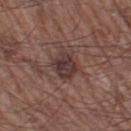Q: Was a biopsy performed?
A: no biopsy performed (imaged during a skin exam)
Q: What is the imaging modality?
A: total-body-photography crop, ~15 mm field of view
Q: What is the anatomic site?
A: the left thigh
Q: What is the lesion's diameter?
A: about 4 mm
Q: What are the patient's age and sex?
A: male, roughly 60 years of age
Q: What lighting was used for the tile?
A: white-light